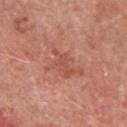Impression:
Part of a total-body skin-imaging series; this lesion was reviewed on a skin check and was not flagged for biopsy.
Acquisition and patient details:
A roughly 15 mm field-of-view crop from a total-body skin photograph. On the chest. The patient is a male approximately 70 years of age. The lesion's longest dimension is about 4 mm. Automated image analysis of the tile measured a border-irregularity rating of about 6.5/10, a within-lesion color-variation index near 0.5/10, and a peripheral color-asymmetry measure near 0. Imaged with white-light lighting.